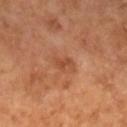Findings:
• biopsy status — catalogued during a skin exam; not biopsied
• image-analysis metrics — a footprint of about 3.5 mm² and a shape eccentricity near 0.8; a detector confidence of about 100 out of 100 that the crop contains a lesion
• tile lighting — cross-polarized illumination
• patient — male, in their mid-60s
• lesion size — ~2.5 mm (longest diameter)
• acquisition — ~15 mm tile from a whole-body skin photo